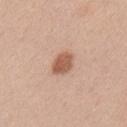The lesion was tiled from a total-body skin photograph and was not biopsied. Measured at roughly 3.5 mm in maximum diameter. The tile uses white-light illumination. The lesion is located on the upper back. Automated image analysis of the tile measured a border-irregularity rating of about 1.5/10, internal color variation of about 2.5 on a 0–10 scale, and peripheral color asymmetry of about 1. A male patient aged 38–42. A 15 mm crop from a total-body photograph taken for skin-cancer surveillance.